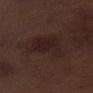imaging modality: 15 mm crop, total-body photography
tile lighting: white-light
patient: male, aged 68 to 72
diameter: about 8 mm
location: the left upper arm
automated metrics: an automated nevus-likeness rating near 10 out of 100 and a detector confidence of about 95 out of 100 that the crop contains a lesion